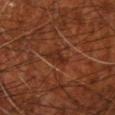Clinical impression: This lesion was catalogued during total-body skin photography and was not selected for biopsy. Background: Automated tile analysis of the lesion measured border irregularity of about 3 on a 0–10 scale and peripheral color asymmetry of about 1.5. The analysis additionally found a nevus-likeness score of about 0/100 and a lesion-detection confidence of about 85/100. Cropped from a total-body skin-imaging series; the visible field is about 15 mm. The tile uses cross-polarized illumination. A male patient, roughly 70 years of age. From the arm.